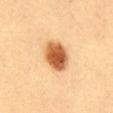This lesion was catalogued during total-body skin photography and was not selected for biopsy.
The subject is a female roughly 30 years of age.
A region of skin cropped from a whole-body photographic capture, roughly 15 mm wide.
The lesion is located on the mid back.
About 4.5 mm across.
Captured under cross-polarized illumination.